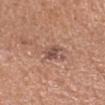Part of a total-body skin-imaging series; this lesion was reviewed on a skin check and was not flagged for biopsy. About 2.5 mm across. The lesion-visualizer software estimated an automated nevus-likeness rating near 0 out of 100 and a lesion-detection confidence of about 100/100. The lesion is located on the right forearm. The tile uses white-light illumination. A male patient, approximately 55 years of age. Cropped from a whole-body photographic skin survey; the tile spans about 15 mm.